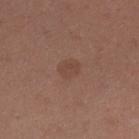Imaged during a routine full-body skin examination; the lesion was not biopsied and no histopathology is available. The lesion is on the right lower leg. Longest diameter approximately 2.5 mm. A female patient aged approximately 30. This is a white-light tile. A lesion tile, about 15 mm wide, cut from a 3D total-body photograph. An algorithmic analysis of the crop reported a border-irregularity rating of about 1.5/10, a within-lesion color-variation index near 1.5/10, and peripheral color asymmetry of about 0.5. The analysis additionally found a lesion-detection confidence of about 100/100.